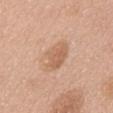Background:
A lesion tile, about 15 mm wide, cut from a 3D total-body photograph. A female patient aged 58–62. Located on the front of the torso. The lesion-visualizer software estimated an average lesion color of about L≈62 a*≈20 b*≈33 (CIELAB) and about 9 CIELAB-L* units darker than the surrounding skin.